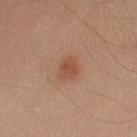The lesion was tiled from a total-body skin photograph and was not biopsied. This image is a 15 mm lesion crop taken from a total-body photograph. A male subject in their 50s. The lesion is on the arm. This is a white-light tile. An algorithmic analysis of the crop reported a classifier nevus-likeness of about 80/100 and a detector confidence of about 100 out of 100 that the crop contains a lesion. The lesion's longest dimension is about 2.5 mm.